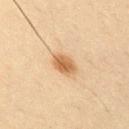Case summary:
• follow-up · imaged on a skin check; not biopsied
• acquisition · ~15 mm crop, total-body skin-cancer survey
• lesion size · about 3.5 mm
• location · the chest
• subject · male, aged approximately 35